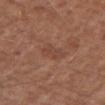Assessment: No biopsy was performed on this lesion — it was imaged during a full skin examination and was not determined to be concerning.